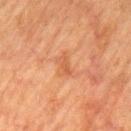The lesion was tiled from a total-body skin photograph and was not biopsied. Located on the mid back. The recorded lesion diameter is about 3 mm. The subject is a male in their 80s. A 15 mm close-up extracted from a 3D total-body photography capture. Automated tile analysis of the lesion measured a footprint of about 3 mm² and an outline eccentricity of about 0.9 (0 = round, 1 = elongated). The analysis additionally found border irregularity of about 4 on a 0–10 scale, a within-lesion color-variation index near 0/10, and a peripheral color-asymmetry measure near 0. The analysis additionally found an automated nevus-likeness rating near 0 out of 100 and a lesion-detection confidence of about 100/100. This is a cross-polarized tile.